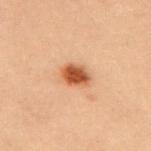This lesion was catalogued during total-body skin photography and was not selected for biopsy. A female subject, aged 28 to 32. Automated tile analysis of the lesion measured an area of roughly 6.5 mm², a shape eccentricity near 0.7, and a symmetry-axis asymmetry near 0.2. Located on the upper back. This is a cross-polarized tile. A 15 mm close-up tile from a total-body photography series done for melanoma screening.